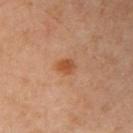Findings:
- workup — catalogued during a skin exam; not biopsied
- lesion diameter — about 2 mm
- lighting — cross-polarized
- patient — male, approximately 55 years of age
- TBP lesion metrics — a mean CIELAB color near L≈51 a*≈26 b*≈38 and a lesion-to-skin contrast of about 8 (normalized; higher = more distinct)
- acquisition — 15 mm crop, total-body photography
- location — the arm